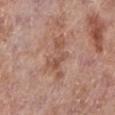No biopsy was performed on this lesion — it was imaged during a full skin examination and was not determined to be concerning. The subject is a female roughly 75 years of age. An algorithmic analysis of the crop reported an area of roughly 10 mm² and two-axis asymmetry of about 0.6. The software also gave a lesion–skin lightness drop of about 8 and a normalized lesion–skin contrast near 6. The software also gave a lesion-detection confidence of about 100/100. Longest diameter approximately 5.5 mm. From the left lower leg. The tile uses white-light illumination. A roughly 15 mm field-of-view crop from a total-body skin photograph.